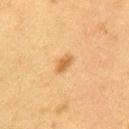| key | value |
|---|---|
| notes | imaged on a skin check; not biopsied |
| acquisition | 15 mm crop, total-body photography |
| anatomic site | the mid back |
| image-analysis metrics | a lesion area of about 3 mm², a shape eccentricity near 0.9, and two-axis asymmetry of about 0.2; border irregularity of about 2 on a 0–10 scale, a color-variation rating of about 1/10, and peripheral color asymmetry of about 0.5 |
| lesion size | about 2.5 mm |
| patient | female, aged around 40 |